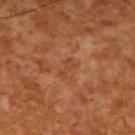Findings:
• workup: imaged on a skin check; not biopsied
• image: ~15 mm crop, total-body skin-cancer survey
• patient: male, aged 63–67